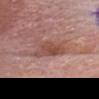Captured during whole-body skin photography for melanoma surveillance; the lesion was not biopsied. This is a white-light tile. Cropped from a whole-body photographic skin survey; the tile spans about 15 mm. A male subject approximately 60 years of age. Located on the head or neck. The lesion-visualizer software estimated a footprint of about 8.5 mm², an outline eccentricity of about 0.85 (0 = round, 1 = elongated), and a shape-asymmetry score of about 0.35 (0 = symmetric). It also reported an average lesion color of about L≈49 a*≈23 b*≈26 (CIELAB), roughly 9 lightness units darker than nearby skin, and a normalized lesion–skin contrast near 7.5. And it measured an automated nevus-likeness rating near 5 out of 100. Longest diameter approximately 5 mm.